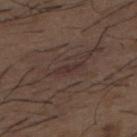Impression:
Captured during whole-body skin photography for melanoma surveillance; the lesion was not biopsied.
Background:
The lesion-visualizer software estimated an area of roughly 2 mm², a shape eccentricity near 0.95, and a symmetry-axis asymmetry near 0.3. And it measured about 6 CIELAB-L* units darker than the surrounding skin and a normalized lesion–skin contrast near 6. It also reported a lesion-detection confidence of about 55/100. The patient is a male aged 48–52. The lesion's longest dimension is about 3 mm. The lesion is on the back. Captured under white-light illumination. Cropped from a total-body skin-imaging series; the visible field is about 15 mm.